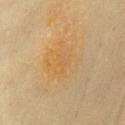Q: What kind of image is this?
A: ~15 mm tile from a whole-body skin photo
Q: Patient demographics?
A: female, aged 58–62
Q: Lesion location?
A: the chest
Q: What is the lesion's diameter?
A: ~4.5 mm (longest diameter)